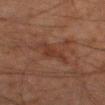lesion diameter = ~4.5 mm (longest diameter) | acquisition = ~15 mm crop, total-body skin-cancer survey | anatomic site = the right thigh | illumination = cross-polarized | patient = male, about 80 years old | automated metrics = a footprint of about 5.5 mm² and a symmetry-axis asymmetry near 0.4; a nevus-likeness score of about 0/100 and a lesion-detection confidence of about 95/100.